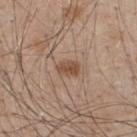No biopsy was performed on this lesion — it was imaged during a full skin examination and was not determined to be concerning. Cropped from a total-body skin-imaging series; the visible field is about 15 mm. The tile uses white-light illumination. On the upper back. The subject is a male roughly 50 years of age. Automated tile analysis of the lesion measured a mean CIELAB color near L≈50 a*≈19 b*≈30, roughly 10 lightness units darker than nearby skin, and a normalized border contrast of about 7.5. The analysis additionally found border irregularity of about 2 on a 0–10 scale, internal color variation of about 2 on a 0–10 scale, and a peripheral color-asymmetry measure near 1. The analysis additionally found a classifier nevus-likeness of about 85/100 and lesion-presence confidence of about 100/100.